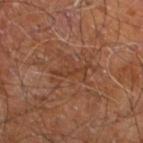The lesion was tiled from a total-body skin photograph and was not biopsied. A male subject, approximately 60 years of age. On the right leg. A 15 mm crop from a total-body photograph taken for skin-cancer surveillance.